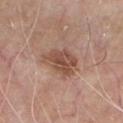Part of a total-body skin-imaging series; this lesion was reviewed on a skin check and was not flagged for biopsy.
The subject is a male aged 78 to 82.
Located on the front of the torso.
A 15 mm crop from a total-body photograph taken for skin-cancer surveillance.